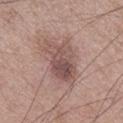Q: Was this lesion biopsied?
A: no biopsy performed (imaged during a skin exam)
Q: How was this image acquired?
A: ~15 mm tile from a whole-body skin photo
Q: Automated lesion metrics?
A: a footprint of about 17 mm², a shape eccentricity near 0.85, and a symmetry-axis asymmetry near 0.35; a lesion color around L≈52 a*≈19 b*≈22 in CIELAB
Q: What is the anatomic site?
A: the leg
Q: What are the patient's age and sex?
A: male, about 50 years old